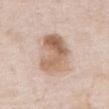<case>
<biopsy_status>not biopsied; imaged during a skin examination</biopsy_status>
<image>
  <source>total-body photography crop</source>
  <field_of_view_mm>15</field_of_view_mm>
</image>
<lesion_size>
  <long_diameter_mm_approx>7.0</long_diameter_mm_approx>
</lesion_size>
<patient>
  <sex>male</sex>
  <age_approx>55</age_approx>
</patient>
<site>chest</site>
<lighting>white-light</lighting>
</case>